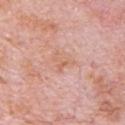Findings:
- follow-up: total-body-photography surveillance lesion; no biopsy
- lesion diameter: about 2.5 mm
- subject: male, aged 78 to 82
- anatomic site: the chest
- TBP lesion metrics: a lesion area of about 3.5 mm² and an eccentricity of roughly 0.45; roughly 6 lightness units darker than nearby skin and a normalized border contrast of about 5.5; a nevus-likeness score of about 0/100 and a lesion-detection confidence of about 100/100
- illumination: white-light illumination
- image: 15 mm crop, total-body photography